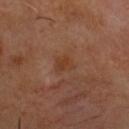Q: Was this lesion biopsied?
A: no biopsy performed (imaged during a skin exam)
Q: What did automated image analysis measure?
A: a detector confidence of about 100 out of 100 that the crop contains a lesion
Q: What kind of image is this?
A: ~15 mm tile from a whole-body skin photo
Q: Where on the body is the lesion?
A: the chest
Q: What lighting was used for the tile?
A: cross-polarized
Q: What is the lesion's diameter?
A: ≈2.5 mm
Q: Patient demographics?
A: male, about 50 years old